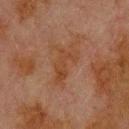{
  "biopsy_status": "not biopsied; imaged during a skin examination",
  "image": {
    "source": "total-body photography crop",
    "field_of_view_mm": 15
  },
  "lesion_size": {
    "long_diameter_mm_approx": 5.0
  },
  "patient": {
    "sex": "male",
    "age_approx": 80
  },
  "automated_metrics": {
    "nevus_likeness_0_100": 0
  },
  "site": "upper back"
}